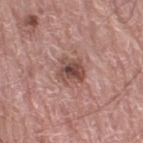* image — 15 mm crop, total-body photography
* lesion size — about 3.5 mm
* patient — male, aged 73–77
* automated metrics — a footprint of about 8 mm², a shape eccentricity near 0.3, and a shape-asymmetry score of about 0.2 (0 = symmetric); border irregularity of about 2.5 on a 0–10 scale and radial color variation of about 2; an automated nevus-likeness rating near 40 out of 100 and a detector confidence of about 100 out of 100 that the crop contains a lesion
* body site — the right thigh
* tile lighting — white-light illumination